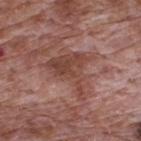{
  "biopsy_status": "not biopsied; imaged during a skin examination",
  "automated_metrics": {
    "border_irregularity_0_10": 7.5,
    "peripheral_color_asymmetry": 1.5
  },
  "patient": {
    "sex": "male",
    "age_approx": 70
  },
  "image": {
    "source": "total-body photography crop",
    "field_of_view_mm": 15
  },
  "site": "back"
}